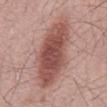<case>
  <biopsy_status>not biopsied; imaged during a skin examination</biopsy_status>
  <site>abdomen</site>
  <lesion_size>
    <long_diameter_mm_approx>9.0</long_diameter_mm_approx>
  </lesion_size>
  <image>
    <source>total-body photography crop</source>
    <field_of_view_mm>15</field_of_view_mm>
  </image>
  <automated_metrics>
    <area_mm2_approx>30.0</area_mm2_approx>
    <eccentricity>0.9</eccentricity>
    <shape_asymmetry>0.15</shape_asymmetry>
    <cielab_L>51</cielab_L>
    <cielab_a>24</cielab_a>
    <cielab_b>24</cielab_b>
    <color_variation_0_10>4.5</color_variation_0_10>
    <peripheral_color_asymmetry>1.5</peripheral_color_asymmetry>
  </automated_metrics>
  <lighting>white-light</lighting>
  <patient>
    <sex>male</sex>
    <age_approx>60</age_approx>
  </patient>
</case>